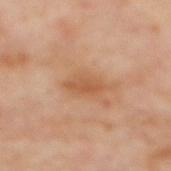Q: Was this lesion biopsied?
A: total-body-photography surveillance lesion; no biopsy